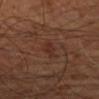Recorded during total-body skin imaging; not selected for excision or biopsy. This image is a 15 mm lesion crop taken from a total-body photograph. The lesion is located on the left forearm. The patient is approximately 65 years of age.